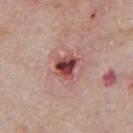notes = total-body-photography surveillance lesion; no biopsy
image = ~15 mm crop, total-body skin-cancer survey
size = ≈3 mm
subject = male, aged 63 to 67
anatomic site = the front of the torso